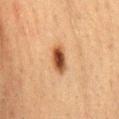{"image": {"source": "total-body photography crop", "field_of_view_mm": 15}, "automated_metrics": {"area_mm2_approx": 5.5, "eccentricity": 0.9, "shape_asymmetry": 0.25, "cielab_L": 41, "cielab_a": 21, "cielab_b": 32, "vs_skin_darker_L": 16.0, "vs_skin_contrast_norm": 12.0, "border_irregularity_0_10": 2.0, "color_variation_0_10": 5.0, "peripheral_color_asymmetry": 1.5, "nevus_likeness_0_100": 100, "lesion_detection_confidence_0_100": 100}, "site": "front of the torso", "lighting": "cross-polarized", "patient": {"sex": "female", "age_approx": 55}, "lesion_size": {"long_diameter_mm_approx": 4.0}}